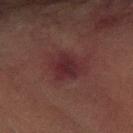This lesion was catalogued during total-body skin photography and was not selected for biopsy. Cropped from a total-body skin-imaging series; the visible field is about 15 mm. About 3.5 mm across. From the left forearm. Automated image analysis of the tile measured an average lesion color of about L≈22 a*≈21 b*≈14 (CIELAB), a lesion–skin lightness drop of about 6, and a lesion-to-skin contrast of about 8 (normalized; higher = more distinct). And it measured a border-irregularity rating of about 2.5/10 and a within-lesion color-variation index near 3.5/10. The software also gave a classifier nevus-likeness of about 0/100 and lesion-presence confidence of about 100/100. A male patient in their mid- to late 70s. Imaged with cross-polarized lighting.